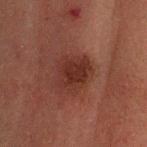No biopsy was performed on this lesion — it was imaged during a full skin examination and was not determined to be concerning. A male patient aged 48 to 52. Cropped from a total-body skin-imaging series; the visible field is about 15 mm. From the head or neck.